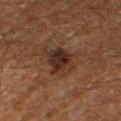Case summary:
- acquisition — ~15 mm crop, total-body skin-cancer survey
- image-analysis metrics — a normalized border contrast of about 9.5; a border-irregularity index near 3.5/10 and peripheral color asymmetry of about 2; a nevus-likeness score of about 70/100 and lesion-presence confidence of about 100/100
- site — the right thigh
- illumination — cross-polarized
- subject — male, in their 60s
- size — about 4.5 mm